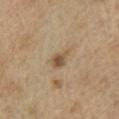| feature | finding |
|---|---|
| biopsy status | catalogued during a skin exam; not biopsied |
| patient | male, in their 70s |
| automated metrics | a lesion area of about 4 mm², an outline eccentricity of about 0.75 (0 = round, 1 = elongated), and a symmetry-axis asymmetry near 0.35; a border-irregularity index near 3/10 |
| location | the left upper arm |
| lesion diameter | ~3 mm (longest diameter) |
| imaging modality | ~15 mm tile from a whole-body skin photo |